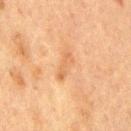biopsy status = total-body-photography surveillance lesion; no biopsy | automated metrics = a border-irregularity index near 3.5/10 and a color-variation rating of about 2/10; a nevus-likeness score of about 0/100 | image = ~15 mm tile from a whole-body skin photo | location = the chest | illumination = cross-polarized illumination | patient = male, in their mid-70s | lesion size = ~4 mm (longest diameter).